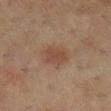{
  "biopsy_status": "not biopsied; imaged during a skin examination",
  "patient": {
    "sex": "female",
    "age_approx": 55
  },
  "image": {
    "source": "total-body photography crop",
    "field_of_view_mm": 15
  },
  "site": "right lower leg"
}